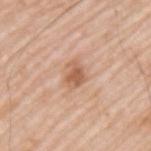biopsy status: no biopsy performed (imaged during a skin exam) | site: the upper back | image source: ~15 mm crop, total-body skin-cancer survey | tile lighting: white-light illumination | lesion size: about 3 mm | patient: male, aged 63–67.